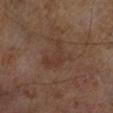Acquisition and patient details: Automated tile analysis of the lesion measured a shape-asymmetry score of about 0.5 (0 = symmetric). The analysis additionally found an average lesion color of about L≈35 a*≈17 b*≈24 (CIELAB), about 5 CIELAB-L* units darker than the surrounding skin, and a normalized border contrast of about 4.5. It also reported a border-irregularity index near 6/10 and a color-variation rating of about 1.5/10. It also reported a detector confidence of about 100 out of 100 that the crop contains a lesion. The lesion's longest dimension is about 4.5 mm. A roughly 15 mm field-of-view crop from a total-body skin photograph. The subject is a male approximately 65 years of age.